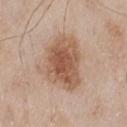  biopsy_status: not biopsied; imaged during a skin examination
  lesion_size:
    long_diameter_mm_approx: 6.5
  image:
    source: total-body photography crop
    field_of_view_mm: 15
  site: chest
  patient:
    sex: male
    age_approx: 65
  automated_metrics:
    area_mm2_approx: 22.0
    eccentricity: 0.75
    shape_asymmetry: 0.25
    cielab_L: 55
    cielab_a: 20
    cielab_b: 31
    vs_skin_darker_L: 12.0
    vs_skin_contrast_norm: 8.5
    border_irregularity_0_10: 3.0
    color_variation_0_10: 5.0
    peripheral_color_asymmetry: 1.5
    nevus_likeness_0_100: 95
    lesion_detection_confidence_0_100: 100
  lighting: white-light